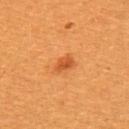biopsy status = total-body-photography surveillance lesion; no biopsy
patient = female, about 55 years old
body site = the upper back
image = ~15 mm tile from a whole-body skin photo